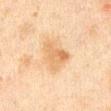Clinical impression:
Recorded during total-body skin imaging; not selected for excision or biopsy.
Acquisition and patient details:
Automated tile analysis of the lesion measured a mean CIELAB color near L≈57 a*≈16 b*≈34, about 8 CIELAB-L* units darker than the surrounding skin, and a normalized border contrast of about 6.5. It also reported a color-variation rating of about 4.5/10. The software also gave a classifier nevus-likeness of about 0/100 and a lesion-detection confidence of about 100/100. A lesion tile, about 15 mm wide, cut from a 3D total-body photograph. The patient is a male aged 43–47. Imaged with cross-polarized lighting. The lesion is located on the chest.